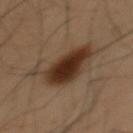The lesion was tiled from a total-body skin photograph and was not biopsied. A male patient aged approximately 55. The lesion is located on the chest. A roughly 15 mm field-of-view crop from a total-body skin photograph. About 6 mm across. This is a cross-polarized tile.